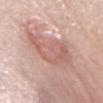The lesion was tiled from a total-body skin photograph and was not biopsied.
The lesion is on the abdomen.
The recorded lesion diameter is about 6.5 mm.
This image is a 15 mm lesion crop taken from a total-body photograph.
An algorithmic analysis of the crop reported a shape eccentricity near 0.85 and a shape-asymmetry score of about 0.4 (0 = symmetric). The software also gave an average lesion color of about L≈61 a*≈22 b*≈25 (CIELAB), about 8 CIELAB-L* units darker than the surrounding skin, and a normalized border contrast of about 5.5.
A female patient in their mid-60s.